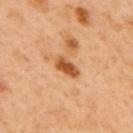Background:
From the mid back. Measured at roughly 3.5 mm in maximum diameter. A male patient approximately 50 years of age. This image is a 15 mm lesion crop taken from a total-body photograph. An algorithmic analysis of the crop reported a footprint of about 5 mm², a shape eccentricity near 0.85, and a shape-asymmetry score of about 0.25 (0 = symmetric). The software also gave a lesion color around L≈54 a*≈26 b*≈42 in CIELAB, roughly 15 lightness units darker than nearby skin, and a normalized lesion–skin contrast near 10. The analysis additionally found border irregularity of about 2.5 on a 0–10 scale, internal color variation of about 3.5 on a 0–10 scale, and radial color variation of about 1. It also reported a classifier nevus-likeness of about 80/100 and a detector confidence of about 100 out of 100 that the crop contains a lesion. Imaged with cross-polarized lighting.